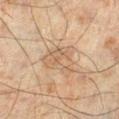– follow-up — total-body-photography surveillance lesion; no biopsy
– body site — the left lower leg
– patient — male, in their mid-40s
– tile lighting — cross-polarized
– acquisition — ~15 mm tile from a whole-body skin photo
– lesion size — about 5 mm